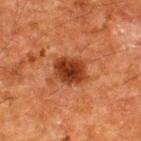patient — male, approximately 60 years of age | diameter — ~4 mm (longest diameter) | automated lesion analysis — border irregularity of about 2 on a 0–10 scale and internal color variation of about 4 on a 0–10 scale; a detector confidence of about 100 out of 100 that the crop contains a lesion | tile lighting — cross-polarized | imaging modality — total-body-photography crop, ~15 mm field of view | body site — the upper back.